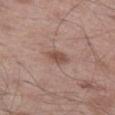This lesion was catalogued during total-body skin photography and was not selected for biopsy. On the left thigh. The tile uses white-light illumination. A 15 mm close-up extracted from a 3D total-body photography capture. A male patient aged approximately 50.